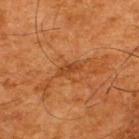| key | value |
|---|---|
| biopsy status | imaged on a skin check; not biopsied |
| image | total-body-photography crop, ~15 mm field of view |
| patient | male, aged 63–67 |
| location | the upper back |